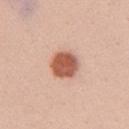Part of a total-body skin-imaging series; this lesion was reviewed on a skin check and was not flagged for biopsy.
The subject is a female aged around 25.
Captured under white-light illumination.
Automated tile analysis of the lesion measured a lesion color around L≈57 a*≈26 b*≈32 in CIELAB, a lesion–skin lightness drop of about 17, and a normalized lesion–skin contrast near 11. It also reported a nevus-likeness score of about 100/100 and lesion-presence confidence of about 100/100.
On the right upper arm.
This image is a 15 mm lesion crop taken from a total-body photograph.